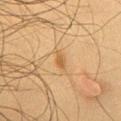{"biopsy_status": "not biopsied; imaged during a skin examination", "site": "chest", "patient": {"sex": "male", "age_approx": 45}, "lighting": "cross-polarized", "image": {"source": "total-body photography crop", "field_of_view_mm": 15}, "lesion_size": {"long_diameter_mm_approx": 2.5}}